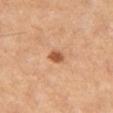Captured during whole-body skin photography for melanoma surveillance; the lesion was not biopsied.
A lesion tile, about 15 mm wide, cut from a 3D total-body photograph.
A female subject about 60 years old.
Approximately 2 mm at its widest.
Located on the leg.